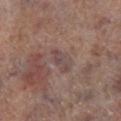Q: Was a biopsy performed?
A: total-body-photography surveillance lesion; no biopsy
Q: What is the anatomic site?
A: the right lower leg
Q: What kind of image is this?
A: ~15 mm tile from a whole-body skin photo
Q: Patient demographics?
A: male, aged around 70